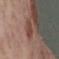Clinical impression:
The lesion was photographed on a routine skin check and not biopsied; there is no pathology result.
Context:
Imaged with cross-polarized lighting. Automated image analysis of the tile measured an average lesion color of about L≈44 a*≈21 b*≈24 (CIELAB), roughly 8 lightness units darker than nearby skin, and a normalized border contrast of about 6.5. The software also gave a color-variation rating of about 2/10 and radial color variation of about 0.5. The recorded lesion diameter is about 4.5 mm. From the right lower leg. A roughly 15 mm field-of-view crop from a total-body skin photograph. A female patient, in their mid-50s.